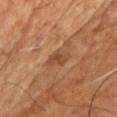Recorded during total-body skin imaging; not selected for excision or biopsy.
A close-up tile cropped from a whole-body skin photograph, about 15 mm across.
From the chest.
The patient is a male aged 53 to 57.
This is a cross-polarized tile.
About 3 mm across.